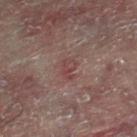This is a cross-polarized tile. The subject is a male about 60 years old. From the leg. A close-up tile cropped from a whole-body skin photograph, about 15 mm across.